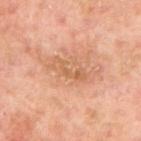The lesion was tiled from a total-body skin photograph and was not biopsied. A 15 mm close-up extracted from a 3D total-body photography capture. A male subject in their 50s. Longest diameter approximately 4.5 mm. This is a cross-polarized tile. The lesion is located on the left arm.